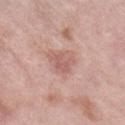Impression: This lesion was catalogued during total-body skin photography and was not selected for biopsy. Image and clinical context: The lesion is located on the right lower leg. Cropped from a whole-body photographic skin survey; the tile spans about 15 mm. Measured at roughly 3 mm in maximum diameter. A female patient, aged 68 to 72.